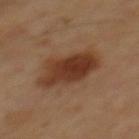Impression: No biopsy was performed on this lesion — it was imaged during a full skin examination and was not determined to be concerning. Clinical summary: Cropped from a whole-body photographic skin survey; the tile spans about 15 mm. The patient is a male in their mid- to late 60s. Located on the mid back. The total-body-photography lesion software estimated an area of roughly 18 mm². It also reported a lesion color around L≈36 a*≈21 b*≈30 in CIELAB, roughly 12 lightness units darker than nearby skin, and a normalized lesion–skin contrast near 10. The software also gave a nevus-likeness score of about 100/100 and lesion-presence confidence of about 100/100. Captured under cross-polarized illumination.